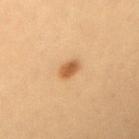{
  "biopsy_status": "not biopsied; imaged during a skin examination",
  "automated_metrics": {
    "area_mm2_approx": 3.5,
    "eccentricity": 0.65,
    "shape_asymmetry": 0.25
  },
  "lesion_size": {
    "long_diameter_mm_approx": 2.5
  },
  "lighting": "cross-polarized",
  "patient": {
    "sex": "female",
    "age_approx": 30
  },
  "site": "right forearm",
  "image": {
    "source": "total-body photography crop",
    "field_of_view_mm": 15
  }
}